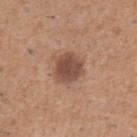| key | value |
|---|---|
| notes | no biopsy performed (imaged during a skin exam) |
| acquisition | ~15 mm tile from a whole-body skin photo |
| site | the right upper arm |
| lighting | white-light illumination |
| lesion diameter | ≈3.5 mm |
| automated lesion analysis | a lesion area of about 9 mm², an outline eccentricity of about 0.45 (0 = round, 1 = elongated), and two-axis asymmetry of about 0.15; a border-irregularity index near 1.5/10 and peripheral color asymmetry of about 0.5; a lesion-detection confidence of about 100/100 |
| subject | male, in their 50s |